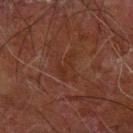This lesion was catalogued during total-body skin photography and was not selected for biopsy.
A lesion tile, about 15 mm wide, cut from a 3D total-body photograph.
The lesion-visualizer software estimated an automated nevus-likeness rating near 0 out of 100.
Approximately 4 mm at its widest.
The lesion is on the arm.
The patient is a male in their 70s.